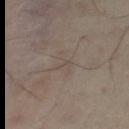- imaging modality: 15 mm crop, total-body photography
- patient: female, in their mid- to late 40s
- anatomic site: the right leg